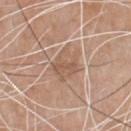Impression:
The lesion was tiled from a total-body skin photograph and was not biopsied.
Image and clinical context:
On the chest. This image is a 15 mm lesion crop taken from a total-body photograph. About 4 mm across. A male patient aged 78–82.